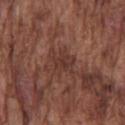This lesion was catalogued during total-body skin photography and was not selected for biopsy. The patient is a male approximately 75 years of age. A roughly 15 mm field-of-view crop from a total-body skin photograph. From the chest. Longest diameter approximately 3 mm. Captured under white-light illumination.